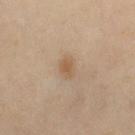| feature | finding |
|---|---|
| lesion diameter | ~2.5 mm (longest diameter) |
| anatomic site | the right thigh |
| patient | female, in their 50s |
| automated metrics | an area of roughly 4 mm², an eccentricity of roughly 0.75, and a shape-asymmetry score of about 0.25 (0 = symmetric); a mean CIELAB color near L≈55 a*≈15 b*≈32, about 7 CIELAB-L* units darker than the surrounding skin, and a lesion-to-skin contrast of about 6.5 (normalized; higher = more distinct); a classifier nevus-likeness of about 35/100 |
| imaging modality | total-body-photography crop, ~15 mm field of view |